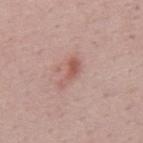Findings:
• workup: catalogued during a skin exam; not biopsied
• diameter: ≈4 mm
• patient: male, aged around 50
• automated lesion analysis: a border-irregularity rating of about 4/10, internal color variation of about 3 on a 0–10 scale, and radial color variation of about 1; a nevus-likeness score of about 15/100 and a lesion-detection confidence of about 100/100
• body site: the upper back
• illumination: white-light illumination
• imaging modality: ~15 mm tile from a whole-body skin photo